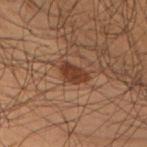Image and clinical context: The subject is a male aged around 55. This is a cross-polarized tile. A roughly 15 mm field-of-view crop from a total-body skin photograph. From the left upper arm.